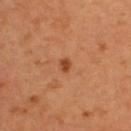Clinical impression:
No biopsy was performed on this lesion — it was imaged during a full skin examination and was not determined to be concerning.
Acquisition and patient details:
A female subject aged approximately 35. A 15 mm close-up tile from a total-body photography series done for melanoma screening. Longest diameter approximately 1.5 mm. The tile uses cross-polarized illumination. Located on the upper back. Automated tile analysis of the lesion measured an area of roughly 2 mm², a shape eccentricity near 0.3, and a symmetry-axis asymmetry near 0.15. And it measured a border-irregularity rating of about 1/10. It also reported a nevus-likeness score of about 65/100 and lesion-presence confidence of about 100/100.